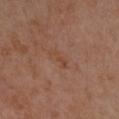The lesion-visualizer software estimated an average lesion color of about L≈45 a*≈21 b*≈30 (CIELAB), roughly 5 lightness units darker than nearby skin, and a normalized border contrast of about 5.5. Imaged with cross-polarized lighting. From the left forearm. Approximately 3 mm at its widest. A 15 mm close-up tile from a total-body photography series done for melanoma screening. The patient is a female aged around 40.